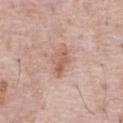Impression:
The lesion was tiled from a total-body skin photograph and was not biopsied.
Image and clinical context:
The subject is a male roughly 70 years of age. A 15 mm close-up extracted from a 3D total-body photography capture. Captured under white-light illumination. From the chest.